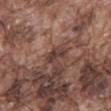| feature | finding |
|---|---|
| workup | imaged on a skin check; not biopsied |
| illumination | white-light illumination |
| image-analysis metrics | border irregularity of about 4.5 on a 0–10 scale, a within-lesion color-variation index near 1/10, and a peripheral color-asymmetry measure near 0.5; an automated nevus-likeness rating near 0 out of 100 and lesion-presence confidence of about 70/100 |
| patient | male, roughly 75 years of age |
| anatomic site | the back |
| lesion size | ~2.5 mm (longest diameter) |
| image | ~15 mm tile from a whole-body skin photo |